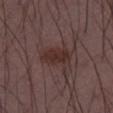Impression: Recorded during total-body skin imaging; not selected for excision or biopsy. Background: The tile uses white-light illumination. An algorithmic analysis of the crop reported a lesion area of about 8 mm² and a symmetry-axis asymmetry near 0.2. The analysis additionally found a border-irregularity rating of about 2/10, internal color variation of about 2.5 on a 0–10 scale, and peripheral color asymmetry of about 1. Cropped from a whole-body photographic skin survey; the tile spans about 15 mm. A male patient aged 48–52. From the left thigh.